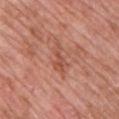Q: Was this lesion biopsied?
A: imaged on a skin check; not biopsied
Q: How was this image acquired?
A: 15 mm crop, total-body photography
Q: Patient demographics?
A: male, aged 48 to 52
Q: Automated lesion metrics?
A: a footprint of about 4 mm², a shape eccentricity near 0.9, and a symmetry-axis asymmetry near 0.45; a lesion–skin lightness drop of about 8
Q: What is the anatomic site?
A: the chest